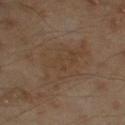biopsy status: catalogued during a skin exam; not biopsied
subject: male, in their mid- to late 40s
imaging modality: 15 mm crop, total-body photography
location: the right upper arm
illumination: cross-polarized